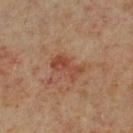<tbp_lesion>
<biopsy_status>not biopsied; imaged during a skin examination</biopsy_status>
<patient>
  <sex>male</sex>
  <age_approx>60</age_approx>
</patient>
<site>left lower leg</site>
<image>
  <source>total-body photography crop</source>
  <field_of_view_mm>15</field_of_view_mm>
</image>
</tbp_lesion>